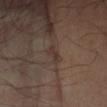Clinical impression: Part of a total-body skin-imaging series; this lesion was reviewed on a skin check and was not flagged for biopsy. Image and clinical context: From the left forearm. Automated image analysis of the tile measured an average lesion color of about L≈32 a*≈14 b*≈19 (CIELAB). The software also gave a border-irregularity index near 4/10, a within-lesion color-variation index near 0/10, and a peripheral color-asymmetry measure near 0. Cropped from a total-body skin-imaging series; the visible field is about 15 mm. A patient roughly 55 years of age. Measured at roughly 2.5 mm in maximum diameter. Imaged with cross-polarized lighting.